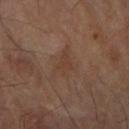The lesion was photographed on a routine skin check and not biopsied; there is no pathology result.
From the right forearm.
Cropped from a whole-body photographic skin survey; the tile spans about 15 mm.
A male patient, aged 83 or older.